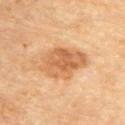Notes:
* workup · no biopsy performed (imaged during a skin exam)
* patient · male, in their mid- to late 80s or older
* location · the upper back
* illumination · cross-polarized illumination
* size · about 6 mm
* imaging modality · ~15 mm tile from a whole-body skin photo